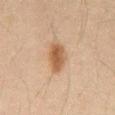follow-up: imaged on a skin check; not biopsied | tile lighting: cross-polarized | image: ~15 mm crop, total-body skin-cancer survey | subject: male, about 65 years old | lesion diameter: about 3.5 mm | automated metrics: a nevus-likeness score of about 100/100 and a detector confidence of about 100 out of 100 that the crop contains a lesion | anatomic site: the front of the torso.